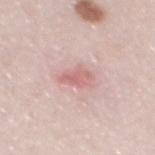notes = imaged on a skin check; not biopsied.